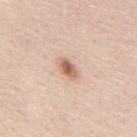<tbp_lesion>
<biopsy_status>not biopsied; imaged during a skin examination</biopsy_status>
<image>
  <source>total-body photography crop</source>
  <field_of_view_mm>15</field_of_view_mm>
</image>
<lesion_size>
  <long_diameter_mm_approx>3.0</long_diameter_mm_approx>
</lesion_size>
<patient>
  <sex>male</sex>
  <age_approx>45</age_approx>
</patient>
<automated_metrics>
  <cielab_L>63</cielab_L>
  <cielab_a>21</cielab_a>
  <cielab_b>30</cielab_b>
  <vs_skin_darker_L>14.0</vs_skin_darker_L>
  <vs_skin_contrast_norm>8.5</vs_skin_contrast_norm>
  <border_irregularity_0_10>2.5</border_irregularity_0_10>
  <color_variation_0_10>4.5</color_variation_0_10>
  <peripheral_color_asymmetry>1.5</peripheral_color_asymmetry>
  <lesion_detection_confidence_0_100>100</lesion_detection_confidence_0_100>
</automated_metrics>
<site>mid back</site>
<lighting>white-light</lighting>
</tbp_lesion>